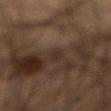biopsy status = no biopsy performed (imaged during a skin exam) | patient = male, about 55 years old | image source = 15 mm crop, total-body photography | location = the abdomen.